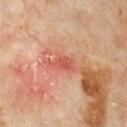| feature | finding |
|---|---|
| imaging modality | ~15 mm crop, total-body skin-cancer survey |
| lesion diameter | ~3 mm (longest diameter) |
| automated metrics | an outline eccentricity of about 0.85 (0 = round, 1 = elongated) and a shape-asymmetry score of about 0.35 (0 = symmetric); a border-irregularity index near 3.5/10, internal color variation of about 3 on a 0–10 scale, and a peripheral color-asymmetry measure near 1; an automated nevus-likeness rating near 0 out of 100 and a lesion-detection confidence of about 100/100 |
| subject | male, aged around 60 |
| tile lighting | cross-polarized illumination |
| body site | the chest |
| diagnosis | a squamous cell carcinoma in situ (malignant) |